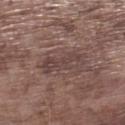follow-up: no biopsy performed (imaged during a skin exam) | image: ~15 mm tile from a whole-body skin photo | anatomic site: the left lower leg | automated lesion analysis: a footprint of about 9 mm², an eccentricity of roughly 0.95, and two-axis asymmetry of about 0.3; a lesion color around L≈42 a*≈17 b*≈18 in CIELAB, about 7 CIELAB-L* units darker than the surrounding skin, and a normalized lesion–skin contrast near 6; a border-irregularity rating of about 4/10, a within-lesion color-variation index near 3/10, and a peripheral color-asymmetry measure near 1; a classifier nevus-likeness of about 0/100 and a detector confidence of about 80 out of 100 that the crop contains a lesion | size: ≈5.5 mm | lighting: white-light | patient: male, approximately 75 years of age.